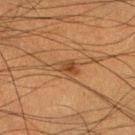Findings:
– subject · male, aged approximately 50
– size · ~3 mm (longest diameter)
– site · the right lower leg
– image · total-body-photography crop, ~15 mm field of view
– lighting · cross-polarized